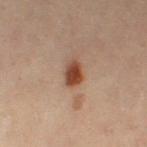{"biopsy_status": "not biopsied; imaged during a skin examination"}